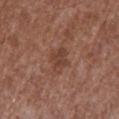Assessment:
Imaged during a routine full-body skin examination; the lesion was not biopsied and no histopathology is available.
Clinical summary:
Cropped from a total-body skin-imaging series; the visible field is about 15 mm. A male patient in their mid- to late 70s. This is a white-light tile. From the mid back.